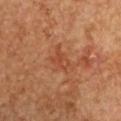follow-up=no biopsy performed (imaged during a skin exam)
illumination=cross-polarized
patient=female, aged approximately 60
image source=15 mm crop, total-body photography
lesion size=~2.5 mm (longest diameter)
anatomic site=the front of the torso
automated lesion analysis=an eccentricity of roughly 0.75 and a symmetry-axis asymmetry near 0.45; a mean CIELAB color near L≈43 a*≈26 b*≈33; a border-irregularity rating of about 4.5/10 and peripheral color asymmetry of about 0.5; a classifier nevus-likeness of about 0/100